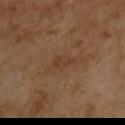Q: Was this lesion biopsied?
A: no biopsy performed (imaged during a skin exam)
Q: Patient demographics?
A: male, aged around 65
Q: What kind of image is this?
A: ~15 mm crop, total-body skin-cancer survey